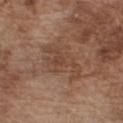Q: Is there a histopathology result?
A: catalogued during a skin exam; not biopsied